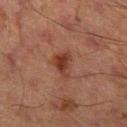Assessment: No biopsy was performed on this lesion — it was imaged during a full skin examination and was not determined to be concerning. Acquisition and patient details: A 15 mm close-up extracted from a 3D total-body photography capture. The lesion is on the right thigh. Automated image analysis of the tile measured border irregularity of about 3.5 on a 0–10 scale, internal color variation of about 4.5 on a 0–10 scale, and peripheral color asymmetry of about 1.5. A male patient, aged around 65. Longest diameter approximately 3.5 mm. Imaged with cross-polarized lighting.